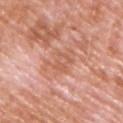Impression:
Part of a total-body skin-imaging series; this lesion was reviewed on a skin check and was not flagged for biopsy.
Context:
Automated image analysis of the tile measured a mean CIELAB color near L≈60 a*≈25 b*≈32. It also reported a classifier nevus-likeness of about 0/100. Captured under white-light illumination. The recorded lesion diameter is about 4.5 mm. A region of skin cropped from a whole-body photographic capture, roughly 15 mm wide. On the front of the torso. A male patient, aged 58–62.